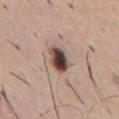workup — no biopsy performed (imaged during a skin exam)
image source — ~15 mm crop, total-body skin-cancer survey
lighting — white-light
subject — male, aged approximately 50
location — the abdomen
automated metrics — an outline eccentricity of about 0.7 (0 = round, 1 = elongated) and two-axis asymmetry of about 0.2; a border-irregularity index near 2/10, internal color variation of about 9.5 on a 0–10 scale, and a peripheral color-asymmetry measure near 3; a detector confidence of about 100 out of 100 that the crop contains a lesion